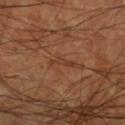Findings:
– follow-up: imaged on a skin check; not biopsied
– patient: male, aged 58 to 62
– acquisition: ~15 mm tile from a whole-body skin photo
– site: the right lower leg